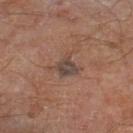No biopsy was performed on this lesion — it was imaged during a full skin examination and was not determined to be concerning.
A 15 mm crop from a total-body photograph taken for skin-cancer surveillance.
A male subject, aged 68–72.
About 3 mm across.
The tile uses cross-polarized illumination.
From the right lower leg.